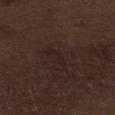Notes:
* notes: no biopsy performed (imaged during a skin exam)
* subject: male, aged 68–72
* location: the left lower leg
* automated lesion analysis: an average lesion color of about L≈19 a*≈13 b*≈16 (CIELAB), roughly 3 lightness units darker than nearby skin, and a normalized lesion–skin contrast near 4.5; a nevus-likeness score of about 0/100 and a detector confidence of about 100 out of 100 that the crop contains a lesion
* lesion diameter: about 3 mm
* imaging modality: 15 mm crop, total-body photography